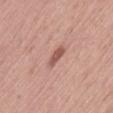Q: Is there a histopathology result?
A: catalogued during a skin exam; not biopsied
Q: How was this image acquired?
A: ~15 mm crop, total-body skin-cancer survey
Q: Lesion location?
A: the leg
Q: What are the patient's age and sex?
A: female, approximately 55 years of age
Q: What did automated image analysis measure?
A: an outline eccentricity of about 0.85 (0 = round, 1 = elongated); a lesion–skin lightness drop of about 12 and a lesion-to-skin contrast of about 8 (normalized; higher = more distinct); a border-irregularity index near 2.5/10, a within-lesion color-variation index near 2/10, and peripheral color asymmetry of about 0.5